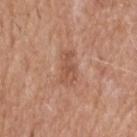No biopsy was performed on this lesion — it was imaged during a full skin examination and was not determined to be concerning. The recorded lesion diameter is about 4 mm. A male patient about 65 years old. On the upper back. A 15 mm close-up tile from a total-body photography series done for melanoma screening. The tile uses white-light illumination.